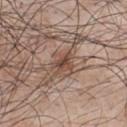The lesion was photographed on a routine skin check and not biopsied; there is no pathology result.
Located on the chest.
A 15 mm close-up extracted from a 3D total-body photography capture.
The subject is a male aged approximately 55.
Imaged with white-light lighting.
About 3.5 mm across.